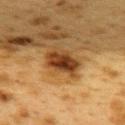patient — female, aged approximately 40; automated lesion analysis — a lesion area of about 8 mm², a shape eccentricity near 0.8, and a shape-asymmetry score of about 0.2 (0 = symmetric); location — the upper back; imaging modality — ~15 mm tile from a whole-body skin photo; pathology — a dysplastic (Clark) nevus.